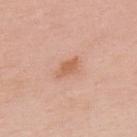Impression: The lesion was photographed on a routine skin check and not biopsied; there is no pathology result. Acquisition and patient details: A male patient, about 50 years old. The lesion is on the upper back. Automated tile analysis of the lesion measured a lesion–skin lightness drop of about 9 and a normalized lesion–skin contrast near 6.5. The software also gave a within-lesion color-variation index near 1.5/10. A roughly 15 mm field-of-view crop from a total-body skin photograph. The recorded lesion diameter is about 3 mm. The tile uses white-light illumination.